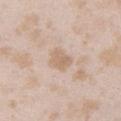<tbp_lesion>
  <biopsy_status>not biopsied; imaged during a skin examination</biopsy_status>
  <automated_metrics>
    <border_irregularity_0_10>2.0</border_irregularity_0_10>
    <color_variation_0_10>2.0</color_variation_0_10>
    <peripheral_color_asymmetry>0.5</peripheral_color_asymmetry>
    <nevus_likeness_0_100>0</nevus_likeness_0_100>
    <lesion_detection_confidence_0_100>100</lesion_detection_confidence_0_100>
  </automated_metrics>
  <patient>
    <sex>female</sex>
    <age_approx>25</age_approx>
  </patient>
  <image>
    <source>total-body photography crop</source>
    <field_of_view_mm>15</field_of_view_mm>
  </image>
  <lesion_size>
    <long_diameter_mm_approx>2.5</long_diameter_mm_approx>
  </lesion_size>
  <site>left upper arm</site>
</tbp_lesion>